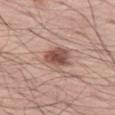Assessment:
No biopsy was performed on this lesion — it was imaged during a full skin examination and was not determined to be concerning.
Background:
Imaged with white-light lighting. A male patient aged 53–57. An algorithmic analysis of the crop reported a lesion area of about 7 mm² and an outline eccentricity of about 0.5 (0 = round, 1 = elongated). The software also gave a color-variation rating of about 4/10 and radial color variation of about 1. A lesion tile, about 15 mm wide, cut from a 3D total-body photograph. About 3 mm across. On the left thigh.